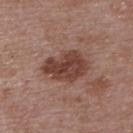notes: imaged on a skin check; not biopsied
acquisition: total-body-photography crop, ~15 mm field of view
illumination: white-light
subject: female, aged 63–67
location: the upper back